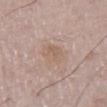This lesion was catalogued during total-body skin photography and was not selected for biopsy.
Imaged with white-light lighting.
A 15 mm close-up extracted from a 3D total-body photography capture.
On the left thigh.
The lesion's longest dimension is about 3.5 mm.
A male subject aged 73–77.